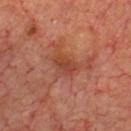<record>
<biopsy_status>not biopsied; imaged during a skin examination</biopsy_status>
<image>
  <source>total-body photography crop</source>
  <field_of_view_mm>15</field_of_view_mm>
</image>
<lesion_size>
  <long_diameter_mm_approx>3.0</long_diameter_mm_approx>
</lesion_size>
<lighting>cross-polarized</lighting>
<patient>
  <sex>male</sex>
  <age_approx>70</age_approx>
</patient>
<site>front of the torso</site>
</record>